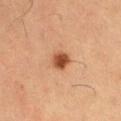size = about 2.5 mm
illumination = cross-polarized
imaging modality = 15 mm crop, total-body photography
subject = male, roughly 60 years of age